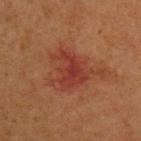workup — imaged on a skin check; not biopsied
site — the upper back
patient — male, aged 63 to 67
illumination — cross-polarized illumination
size — ~5 mm (longest diameter)
imaging modality — ~15 mm crop, total-body skin-cancer survey
automated lesion analysis — a lesion area of about 12 mm², an outline eccentricity of about 0.4 (0 = round, 1 = elongated), and a shape-asymmetry score of about 0.5 (0 = symmetric); a mean CIELAB color near L≈33 a*≈26 b*≈26, roughly 7 lightness units darker than nearby skin, and a lesion-to-skin contrast of about 7 (normalized; higher = more distinct); a classifier nevus-likeness of about 0/100 and a detector confidence of about 100 out of 100 that the crop contains a lesion